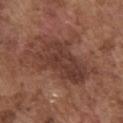notes = catalogued during a skin exam; not biopsied | lighting = white-light | TBP lesion metrics = an average lesion color of about L≈38 a*≈21 b*≈25 (CIELAB), about 9 CIELAB-L* units darker than the surrounding skin, and a normalized border contrast of about 7.5 | diameter = ~9.5 mm (longest diameter) | body site = the chest | subject = male, aged 73–77 | acquisition = total-body-photography crop, ~15 mm field of view.